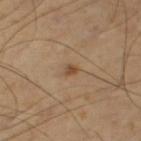This lesion was catalogued during total-body skin photography and was not selected for biopsy. From the leg. A close-up tile cropped from a whole-body skin photograph, about 15 mm across. The patient is a male aged 53 to 57.